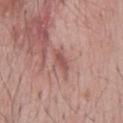The lesion was photographed on a routine skin check and not biopsied; there is no pathology result.
Located on the mid back.
A 15 mm crop from a total-body photograph taken for skin-cancer surveillance.
The patient is a male aged 63 to 67.
Captured under white-light illumination.